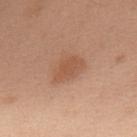The lesion was photographed on a routine skin check and not biopsied; there is no pathology result. The lesion-visualizer software estimated an area of roughly 7.5 mm², an outline eccentricity of about 0.8 (0 = round, 1 = elongated), and two-axis asymmetry of about 0.25. The tile uses white-light illumination. The subject is a male aged 68 to 72. A 15 mm crop from a total-body photograph taken for skin-cancer surveillance. About 4 mm across. The lesion is on the upper back.